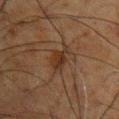Impression: The lesion was photographed on a routine skin check and not biopsied; there is no pathology result. Acquisition and patient details: Automated image analysis of the tile measured a shape eccentricity near 0.85 and a shape-asymmetry score of about 0.35 (0 = symmetric). From the chest. The patient is a male aged approximately 60. A 15 mm close-up tile from a total-body photography series done for melanoma screening.